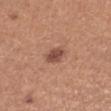Q: How was this image acquired?
A: ~15 mm tile from a whole-body skin photo
Q: What is the lesion's diameter?
A: about 3 mm
Q: What is the anatomic site?
A: the arm
Q: Patient demographics?
A: female, in their mid-20s